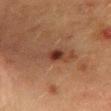Q: Is there a histopathology result?
A: no biopsy performed (imaged during a skin exam)
Q: What is the lesion's diameter?
A: ≈6 mm
Q: What is the imaging modality?
A: ~15 mm crop, total-body skin-cancer survey
Q: Illumination type?
A: cross-polarized
Q: What is the anatomic site?
A: the abdomen
Q: What are the patient's age and sex?
A: female, aged approximately 50